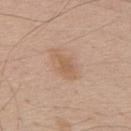No biopsy was performed on this lesion — it was imaged during a full skin examination and was not determined to be concerning.
From the chest.
The patient is a male aged 58 to 62.
A roughly 15 mm field-of-view crop from a total-body skin photograph.